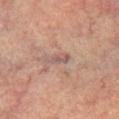Case summary:
• size — ~3 mm (longest diameter)
• image — ~15 mm tile from a whole-body skin photo
• body site — the right lower leg
• subject — male, aged 73 to 77
• illumination — cross-polarized